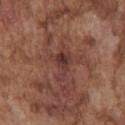Impression:
No biopsy was performed on this lesion — it was imaged during a full skin examination and was not determined to be concerning.
Context:
The lesion is on the chest. Captured under white-light illumination. A roughly 15 mm field-of-view crop from a total-body skin photograph. Automated tile analysis of the lesion measured a footprint of about 7.5 mm² and a shape eccentricity near 0.8. It also reported a mean CIELAB color near L≈37 a*≈22 b*≈22, a lesion–skin lightness drop of about 8, and a normalized border contrast of about 7.5. The recorded lesion diameter is about 4 mm. A male patient aged around 75.